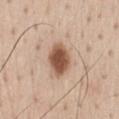The lesion's longest dimension is about 4 mm. Cropped from a whole-body photographic skin survey; the tile spans about 15 mm. From the chest. The tile uses white-light illumination. The lesion-visualizer software estimated an area of roughly 9 mm², a shape eccentricity near 0.75, and a shape-asymmetry score of about 0.15 (0 = symmetric). The software also gave a border-irregularity rating of about 1.5/10, a within-lesion color-variation index near 3.5/10, and a peripheral color-asymmetry measure near 1. It also reported a classifier nevus-likeness of about 100/100 and a detector confidence of about 100 out of 100 that the crop contains a lesion. A male subject, in their mid- to late 40s.